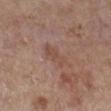workup = total-body-photography surveillance lesion; no biopsy
image = ~15 mm crop, total-body skin-cancer survey
diameter = ≈4 mm
body site = the right lower leg
automated lesion analysis = a footprint of about 6 mm² and a symmetry-axis asymmetry near 0.25; a lesion color around L≈49 a*≈19 b*≈26 in CIELAB, roughly 6 lightness units darker than nearby skin, and a normalized lesion–skin contrast near 5; an automated nevus-likeness rating near 0 out of 100 and lesion-presence confidence of about 100/100
subject = female, aged around 65
tile lighting = white-light illumination